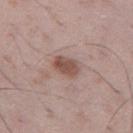Q: Was a biopsy performed?
A: imaged on a skin check; not biopsied
Q: Lesion size?
A: about 3.5 mm
Q: Illumination type?
A: white-light illumination
Q: Patient demographics?
A: male, about 50 years old
Q: Where on the body is the lesion?
A: the leg
Q: How was this image acquired?
A: ~15 mm tile from a whole-body skin photo